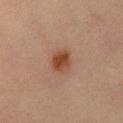Q: Was this lesion biopsied?
A: catalogued during a skin exam; not biopsied
Q: Patient demographics?
A: male, aged 63 to 67
Q: What is the lesion's diameter?
A: ~3.5 mm (longest diameter)
Q: Where on the body is the lesion?
A: the chest
Q: What kind of image is this?
A: 15 mm crop, total-body photography
Q: How was the tile lit?
A: cross-polarized illumination